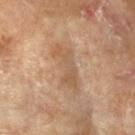Clinical impression:
No biopsy was performed on this lesion — it was imaged during a full skin examination and was not determined to be concerning.
Image and clinical context:
Automated image analysis of the tile measured an area of roughly 9.5 mm² and an outline eccentricity of about 0.95 (0 = round, 1 = elongated). The analysis additionally found a lesion color around L≈57 a*≈18 b*≈33 in CIELAB, a lesion–skin lightness drop of about 8, and a lesion-to-skin contrast of about 5.5 (normalized; higher = more distinct). The software also gave a border-irregularity rating of about 6.5/10 and peripheral color asymmetry of about 1. The lesion's longest dimension is about 6.5 mm. Imaged with cross-polarized lighting. A 15 mm close-up tile from a total-body photography series done for melanoma screening. The lesion is located on the left forearm. A female patient aged 73–77.